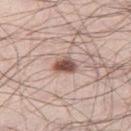<tbp_lesion>
  <biopsy_status>not biopsied; imaged during a skin examination</biopsy_status>
  <lesion_size>
    <long_diameter_mm_approx>3.0</long_diameter_mm_approx>
  </lesion_size>
  <site>right thigh</site>
  <lighting>white-light</lighting>
  <patient>
    <sex>male</sex>
    <age_approx>35</age_approx>
  </patient>
  <image>
    <source>total-body photography crop</source>
    <field_of_view_mm>15</field_of_view_mm>
  </image>
</tbp_lesion>